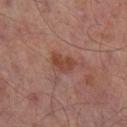Part of a total-body skin-imaging series; this lesion was reviewed on a skin check and was not flagged for biopsy. Cropped from a whole-body photographic skin survey; the tile spans about 15 mm. Located on the leg. A male patient roughly 65 years of age. The lesion-visualizer software estimated a mean CIELAB color near L≈41 a*≈22 b*≈26, a lesion–skin lightness drop of about 8, and a normalized lesion–skin contrast near 7.5. The software also gave border irregularity of about 3 on a 0–10 scale, a within-lesion color-variation index near 2.5/10, and peripheral color asymmetry of about 1. This is a cross-polarized tile.